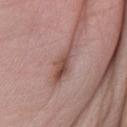Impression:
Captured during whole-body skin photography for melanoma surveillance; the lesion was not biopsied.
Image and clinical context:
Captured under white-light illumination. The lesion is located on the arm. The patient is a female aged 53–57. A region of skin cropped from a whole-body photographic capture, roughly 15 mm wide. Approximately 5.5 mm at its widest.